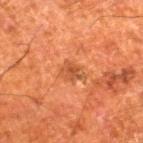Part of a total-body skin-imaging series; this lesion was reviewed on a skin check and was not flagged for biopsy.